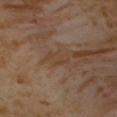tile lighting = cross-polarized illumination
image = 15 mm crop, total-body photography
subject = female, aged 53 to 57
automated lesion analysis = a lesion color around L≈42 a*≈17 b*≈29 in CIELAB; a color-variation rating of about 1/10 and a peripheral color-asymmetry measure near 0.5; a nevus-likeness score of about 0/100 and a detector confidence of about 55 out of 100 that the crop contains a lesion
site = the chest
lesion diameter = ≈3 mm